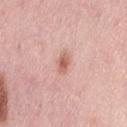notes=imaged on a skin check; not biopsied | illumination=white-light illumination | body site=the lower back | image source=15 mm crop, total-body photography | subject=female, aged around 50.